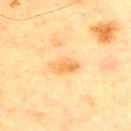notes=imaged on a skin check; not biopsied
image=~15 mm crop, total-body skin-cancer survey
subject=male, in their mid-60s
body site=the chest